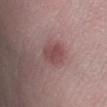{"biopsy_status": "not biopsied; imaged during a skin examination", "image": {"source": "total-body photography crop", "field_of_view_mm": 15}, "patient": {"sex": "male", "age_approx": 50}, "site": "right lower leg"}